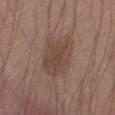Captured during whole-body skin photography for melanoma surveillance; the lesion was not biopsied.
The subject is a male aged 43 to 47.
About 5.5 mm across.
A region of skin cropped from a whole-body photographic capture, roughly 15 mm wide.
On the arm.
Captured under white-light illumination.